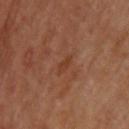A 15 mm crop from a total-body photograph taken for skin-cancer surveillance. A male patient, aged 68–72. The lesion is located on the upper back.